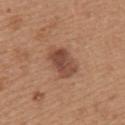Impression: The lesion was photographed on a routine skin check and not biopsied; there is no pathology result. Image and clinical context: The total-body-photography lesion software estimated an average lesion color of about L≈47 a*≈22 b*≈29 (CIELAB), about 11 CIELAB-L* units darker than the surrounding skin, and a normalized lesion–skin contrast near 8.5. It also reported a nevus-likeness score of about 70/100 and a lesion-detection confidence of about 100/100. A 15 mm crop from a total-body photograph taken for skin-cancer surveillance. The patient is a female roughly 35 years of age. About 4 mm across. The lesion is on the upper back. This is a white-light tile.